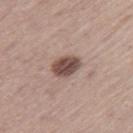notes: total-body-photography surveillance lesion; no biopsy
diameter: about 3.5 mm
lighting: white-light illumination
automated metrics: border irregularity of about 1.5 on a 0–10 scale, a color-variation rating of about 4.5/10, and radial color variation of about 1.5; lesion-presence confidence of about 100/100
image source: 15 mm crop, total-body photography
subject: male, aged around 65
location: the right thigh